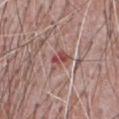The lesion was photographed on a routine skin check and not biopsied; there is no pathology result. The subject is a male in their 70s. A 15 mm close-up extracted from a 3D total-body photography capture. Located on the chest.